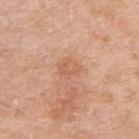biopsy status=total-body-photography surveillance lesion; no biopsy | patient=male, about 65 years old | body site=the right upper arm | image=15 mm crop, total-body photography | tile lighting=white-light illumination | size=about 2.5 mm.